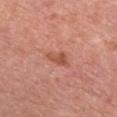Findings:
• notes — total-body-photography surveillance lesion; no biopsy
• automated metrics — an area of roughly 3.5 mm², an outline eccentricity of about 0.85 (0 = round, 1 = elongated), and two-axis asymmetry of about 0.4; a border-irregularity rating of about 4/10, internal color variation of about 1 on a 0–10 scale, and a peripheral color-asymmetry measure near 0.5
• lesion size — about 3 mm
• lighting — white-light illumination
• image source — 15 mm crop, total-body photography
• subject — female, aged 58–62
• body site — the chest